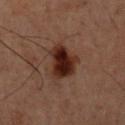workup: total-body-photography surveillance lesion; no biopsy
diameter: about 5 mm
anatomic site: the back
patient: male, roughly 60 years of age
illumination: cross-polarized illumination
imaging modality: 15 mm crop, total-body photography